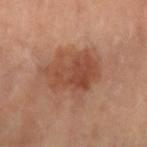Impression: The lesion was tiled from a total-body skin photograph and was not biopsied. Acquisition and patient details: Automated image analysis of the tile measured a lesion-detection confidence of about 100/100. A 15 mm crop from a total-body photograph taken for skin-cancer surveillance. The lesion's longest dimension is about 6.5 mm. The tile uses cross-polarized illumination. The lesion is on the left forearm. A female patient, aged 58 to 62.